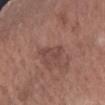Imaged during a routine full-body skin examination; the lesion was not biopsied and no histopathology is available.
Imaged with white-light lighting.
About 3.5 mm across.
The lesion is on the right forearm.
The subject is a male aged 53–57.
A lesion tile, about 15 mm wide, cut from a 3D total-body photograph.